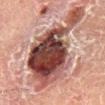Part of a total-body skin-imaging series; this lesion was reviewed on a skin check and was not flagged for biopsy. A roughly 15 mm field-of-view crop from a total-body skin photograph. The subject is a male about 55 years old. Imaged with cross-polarized lighting. The lesion is on the right lower leg. Measured at roughly 11 mm in maximum diameter.